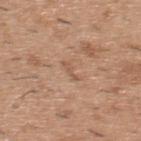Notes:
- biopsy status — imaged on a skin check; not biopsied
- illumination — white-light
- acquisition — total-body-photography crop, ~15 mm field of view
- lesion size — about 2.5 mm
- anatomic site — the upper back
- patient — male, approximately 40 years of age